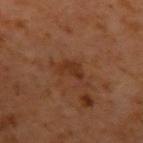Acquisition and patient details: The lesion is on the left upper arm. A male subject approximately 60 years of age. About 5.5 mm across. The tile uses cross-polarized illumination. A close-up tile cropped from a whole-body skin photograph, about 15 mm across.